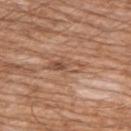illumination: white-light
subject: male, about 60 years old
lesion size: ≈4 mm
image: 15 mm crop, total-body photography
body site: the chest